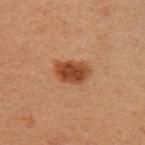Notes:
- biopsy status · no biopsy performed (imaged during a skin exam)
- lighting · cross-polarized illumination
- site · the chest
- automated lesion analysis · a footprint of about 8.5 mm², a shape eccentricity near 0.75, and two-axis asymmetry of about 0.2; a border-irregularity rating of about 2/10, a within-lesion color-variation index near 3/10, and a peripheral color-asymmetry measure near 1
- subject · female, aged approximately 50
- image · ~15 mm crop, total-body skin-cancer survey
- lesion diameter · about 4 mm